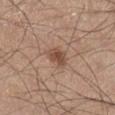Case summary:
- notes · imaged on a skin check; not biopsied
- imaging modality · 15 mm crop, total-body photography
- anatomic site · the right lower leg
- lesion size · ≈3 mm
- patient · male, in their mid-40s
- lighting · white-light illumination